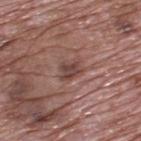Imaged during a routine full-body skin examination; the lesion was not biopsied and no histopathology is available. The tile uses white-light illumination. A male subject approximately 70 years of age. The recorded lesion diameter is about 3 mm. A lesion tile, about 15 mm wide, cut from a 3D total-body photograph. Located on the upper back.